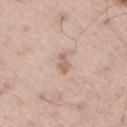No biopsy was performed on this lesion — it was imaged during a full skin examination and was not determined to be concerning. A lesion tile, about 15 mm wide, cut from a 3D total-body photograph. From the right thigh. A male patient aged 53 to 57.